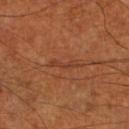<case>
  <biopsy_status>not biopsied; imaged during a skin examination</biopsy_status>
  <automated_metrics>
    <eccentricity>0.95</eccentricity>
    <shape_asymmetry>0.5</shape_asymmetry>
    <cielab_L>38</cielab_L>
    <cielab_a>24</cielab_a>
    <cielab_b>32</cielab_b>
    <vs_skin_darker_L>6.0</vs_skin_darker_L>
    <vs_skin_contrast_norm>5.0</vs_skin_contrast_norm>
  </automated_metrics>
  <image>
    <source>total-body photography crop</source>
    <field_of_view_mm>15</field_of_view_mm>
  </image>
  <lesion_size>
    <long_diameter_mm_approx>3.5</long_diameter_mm_approx>
  </lesion_size>
  <site>leg</site>
  <lighting>cross-polarized</lighting>
  <patient>
    <sex>male</sex>
    <age_approx>70</age_approx>
  </patient>
</case>